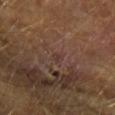Impression: No biopsy was performed on this lesion — it was imaged during a full skin examination and was not determined to be concerning. Clinical summary: Cropped from a total-body skin-imaging series; the visible field is about 15 mm. On the arm. A male patient, approximately 70 years of age. Captured under cross-polarized illumination. Approximately 1.5 mm at its widest.